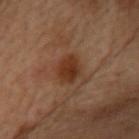Captured during whole-body skin photography for melanoma surveillance; the lesion was not biopsied. The lesion is on the head or neck. Cropped from a total-body skin-imaging series; the visible field is about 15 mm. Measured at roughly 3.5 mm in maximum diameter. A female subject aged 58 to 62.